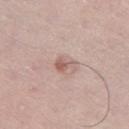Findings:
• follow-up · no biopsy performed (imaged during a skin exam)
• patient · male, approximately 40 years of age
• diameter · ~2.5 mm (longest diameter)
• image · 15 mm crop, total-body photography
• anatomic site · the left thigh
• automated lesion analysis · an average lesion color of about L≈59 a*≈19 b*≈23 (CIELAB) and a normalized lesion–skin contrast near 6.5